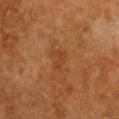notes = no biopsy performed (imaged during a skin exam)
patient = female, aged around 50
TBP lesion metrics = an automated nevus-likeness rating near 0 out of 100 and lesion-presence confidence of about 100/100
imaging modality = ~15 mm crop, total-body skin-cancer survey
anatomic site = the chest
tile lighting = cross-polarized illumination